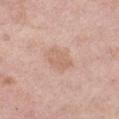{"biopsy_status": "not biopsied; imaged during a skin examination", "automated_metrics": {"color_variation_0_10": 2.0, "peripheral_color_asymmetry": 0.5}, "patient": {"sex": "female", "age_approx": 50}, "site": "left thigh", "image": {"source": "total-body photography crop", "field_of_view_mm": 15}}